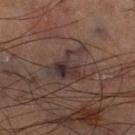Findings:
• follow-up: total-body-photography surveillance lesion; no biopsy
• location: the right thigh
• image: ~15 mm crop, total-body skin-cancer survey